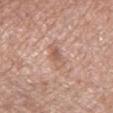Recorded during total-body skin imaging; not selected for excision or biopsy. The patient is a female in their 70s. A lesion tile, about 15 mm wide, cut from a 3D total-body photograph. About 4 mm across. From the right forearm. This is a white-light tile. Automated image analysis of the tile measured an outline eccentricity of about 0.9 (0 = round, 1 = elongated) and a symmetry-axis asymmetry near 0.3. It also reported a lesion color around L≈59 a*≈20 b*≈28 in CIELAB, roughly 8 lightness units darker than nearby skin, and a lesion-to-skin contrast of about 6 (normalized; higher = more distinct). The software also gave border irregularity of about 3 on a 0–10 scale and a peripheral color-asymmetry measure near 1.5. The software also gave a nevus-likeness score of about 0/100.